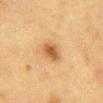Findings:
* imaging modality · ~15 mm crop, total-body skin-cancer survey
* patient · female, roughly 55 years of age
* tile lighting · cross-polarized
* size · ~2.5 mm (longest diameter)
* location · the front of the torso